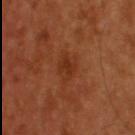Q: Was this lesion biopsied?
A: catalogued during a skin exam; not biopsied